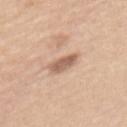notes: catalogued during a skin exam; not biopsied
anatomic site: the mid back
imaging modality: total-body-photography crop, ~15 mm field of view
patient: female, aged 63–67